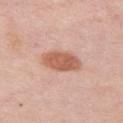Findings:
– follow-up — catalogued during a skin exam; not biopsied
– automated lesion analysis — an area of roughly 10 mm² and an outline eccentricity of about 0.85 (0 = round, 1 = elongated); a border-irregularity rating of about 2/10 and internal color variation of about 3 on a 0–10 scale; an automated nevus-likeness rating near 100 out of 100 and lesion-presence confidence of about 100/100
– imaging modality — ~15 mm tile from a whole-body skin photo
– patient — female, roughly 65 years of age
– lighting — white-light illumination
– site — the chest
– diameter — ≈4.5 mm